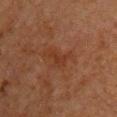biopsy status: no biopsy performed (imaged during a skin exam) | acquisition: 15 mm crop, total-body photography | TBP lesion metrics: an area of roughly 2.5 mm², an eccentricity of roughly 0.9, and a shape-asymmetry score of about 0.5 (0 = symmetric); roughly 5 lightness units darker than nearby skin and a normalized border contrast of about 5.5; a color-variation rating of about 0/10 and a peripheral color-asymmetry measure near 0; a classifier nevus-likeness of about 0/100 | diameter: ≈2.5 mm | subject: male, aged 58 to 62 | site: the chest.